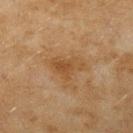Recorded during total-body skin imaging; not selected for excision or biopsy.
From the arm.
The subject is a female about 60 years old.
Cropped from a total-body skin-imaging series; the visible field is about 15 mm.